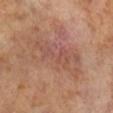* follow-up — imaged on a skin check; not biopsied
* patient — male, approximately 65 years of age
* automated lesion analysis — a border-irregularity rating of about 5/10, internal color variation of about 4.5 on a 0–10 scale, and radial color variation of about 1.5; an automated nevus-likeness rating near 0 out of 100
* tile lighting — cross-polarized illumination
* image source — ~15 mm crop, total-body skin-cancer survey